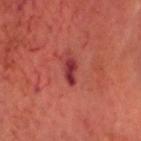The lesion was tiled from a total-body skin photograph and was not biopsied.
Automated image analysis of the tile measured about 12 CIELAB-L* units darker than the surrounding skin. It also reported a border-irregularity rating of about 3.5/10, a within-lesion color-variation index near 1/10, and radial color variation of about 0.5.
A roughly 15 mm field-of-view crop from a total-body skin photograph.
A male subject aged approximately 65.
On the head or neck.
The lesion's longest dimension is about 3 mm.
The tile uses cross-polarized illumination.